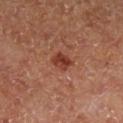The subject is female.
About 2.5 mm across.
A close-up tile cropped from a whole-body skin photograph, about 15 mm across.
Automated image analysis of the tile measured an area of roughly 4.5 mm², an eccentricity of roughly 0.6, and two-axis asymmetry of about 0.3. The analysis additionally found a classifier nevus-likeness of about 90/100.
The lesion is on the right lower leg.
Captured under cross-polarized illumination.